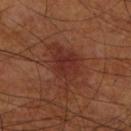| field | value |
|---|---|
| biopsy status | total-body-photography surveillance lesion; no biopsy |
| location | the right lower leg |
| size | about 6.5 mm |
| tile lighting | cross-polarized illumination |
| patient | male, aged 68–72 |
| image source | 15 mm crop, total-body photography |